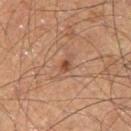<tbp_lesion>
  <image>
    <source>total-body photography crop</source>
    <field_of_view_mm>15</field_of_view_mm>
  </image>
  <lesion_size>
    <long_diameter_mm_approx>1.5</long_diameter_mm_approx>
  </lesion_size>
  <patient>
    <sex>male</sex>
    <age_approx>60</age_approx>
  </patient>
  <site>left thigh</site>
</tbp_lesion>